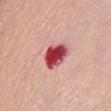biopsy_status: not biopsied; imaged during a skin examination
site: chest
image:
  source: total-body photography crop
  field_of_view_mm: 15
patient:
  sex: female
  age_approx: 65
lighting: white-light
lesion_size:
  long_diameter_mm_approx: 4.0
automated_metrics:
  cielab_L: 50
  cielab_a: 40
  cielab_b: 25
  vs_skin_darker_L: 22.0
  vs_skin_contrast_norm: 14.0
  nevus_likeness_0_100: 0
  lesion_detection_confidence_0_100: 100